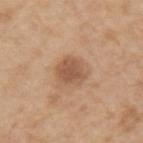A region of skin cropped from a whole-body photographic capture, roughly 15 mm wide. The tile uses white-light illumination. A male subject aged around 70. The lesion is located on the right upper arm. An algorithmic analysis of the crop reported an area of roughly 7.5 mm² and a shape-asymmetry score of about 0.2 (0 = symmetric). The analysis additionally found lesion-presence confidence of about 100/100.